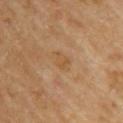Clinical impression:
Imaged during a routine full-body skin examination; the lesion was not biopsied and no histopathology is available.
Context:
About 3 mm across. A female subject, aged approximately 70. The tile uses cross-polarized illumination. From the upper back. Cropped from a whole-body photographic skin survey; the tile spans about 15 mm. The lesion-visualizer software estimated a lesion area of about 4 mm², an outline eccentricity of about 0.75 (0 = round, 1 = elongated), and a symmetry-axis asymmetry near 0.3. The analysis additionally found a lesion color around L≈55 a*≈19 b*≈38 in CIELAB, a lesion–skin lightness drop of about 6, and a lesion-to-skin contrast of about 5 (normalized; higher = more distinct). The analysis additionally found a border-irregularity rating of about 3/10, a within-lesion color-variation index near 2/10, and a peripheral color-asymmetry measure near 0.5.